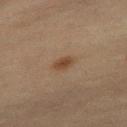patient — female, aged around 45
image — 15 mm crop, total-body photography
diameter — ~2.5 mm (longest diameter)
location — the right thigh